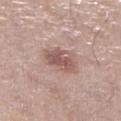Clinical impression:
Imaged during a routine full-body skin examination; the lesion was not biopsied and no histopathology is available.
Context:
The lesion's longest dimension is about 4 mm. This image is a 15 mm lesion crop taken from a total-body photograph. The lesion is located on the left lower leg. The patient is a female in their mid-60s. Captured under white-light illumination.